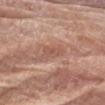Recorded during total-body skin imaging; not selected for excision or biopsy.
The tile uses white-light illumination.
The subject is a male aged 78–82.
On the right upper arm.
A 15 mm crop from a total-body photograph taken for skin-cancer surveillance.